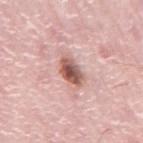The lesion was photographed on a routine skin check and not biopsied; there is no pathology result.
From the back.
A male patient about 75 years old.
A close-up tile cropped from a whole-body skin photograph, about 15 mm across.